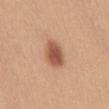biopsy status — total-body-photography surveillance lesion; no biopsy | site — the abdomen | TBP lesion metrics — about 14 CIELAB-L* units darker than the surrounding skin and a lesion-to-skin contrast of about 9 (normalized; higher = more distinct); border irregularity of about 1.5 on a 0–10 scale, a within-lesion color-variation index near 4/10, and peripheral color asymmetry of about 1 | patient — male, about 35 years old | imaging modality — 15 mm crop, total-body photography | lighting — white-light illumination | size — ~3.5 mm (longest diameter).